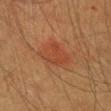Clinical impression: Recorded during total-body skin imaging; not selected for excision or biopsy. Image and clinical context: The tile uses cross-polarized illumination. This image is a 15 mm lesion crop taken from a total-body photograph. Automated image analysis of the tile measured an area of roughly 8 mm² and an outline eccentricity of about 0.65 (0 = round, 1 = elongated). And it measured an average lesion color of about L≈36 a*≈23 b*≈30 (CIELAB) and roughly 5 lightness units darker than nearby skin. It also reported border irregularity of about 3 on a 0–10 scale, a color-variation rating of about 3/10, and peripheral color asymmetry of about 1. The lesion is on the head or neck. The subject is a female in their mid- to late 50s.